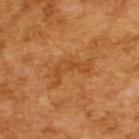Q: Was a biopsy performed?
A: total-body-photography surveillance lesion; no biopsy
Q: How was this image acquired?
A: total-body-photography crop, ~15 mm field of view
Q: Patient demographics?
A: male, aged approximately 65